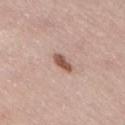No biopsy was performed on this lesion — it was imaged during a full skin examination and was not determined to be concerning. A female patient, aged approximately 50. Captured under white-light illumination. A 15 mm close-up extracted from a 3D total-body photography capture. Located on the right thigh. Longest diameter approximately 2.5 mm.